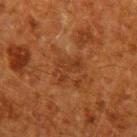Part of a total-body skin-imaging series; this lesion was reviewed on a skin check and was not flagged for biopsy. Measured at roughly 4 mm in maximum diameter. The lesion is on the right upper arm. A 15 mm close-up extracted from a 3D total-body photography capture. A male subject approximately 60 years of age. Imaged with cross-polarized lighting.